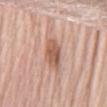Imaged during a routine full-body skin examination; the lesion was not biopsied and no histopathology is available.
A female patient, aged around 65.
Located on the front of the torso.
A roughly 15 mm field-of-view crop from a total-body skin photograph.